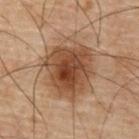Q: What is the anatomic site?
A: the chest
Q: What kind of image is this?
A: 15 mm crop, total-body photography
Q: Patient demographics?
A: male, aged 68–72